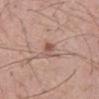| key | value |
|---|---|
| diameter | about 2.5 mm |
| image source | ~15 mm crop, total-body skin-cancer survey |
| subject | male, in their mid-50s |
| site | the mid back |
| TBP lesion metrics | a lesion area of about 2.5 mm², an eccentricity of roughly 0.85, and a symmetry-axis asymmetry near 0.35; a color-variation rating of about 1/10 and radial color variation of about 0 |